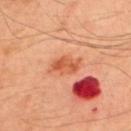workup: no biopsy performed (imaged during a skin exam); illumination: cross-polarized illumination; body site: the upper back; subject: male, aged approximately 50; image-analysis metrics: an area of roughly 6.5 mm² and a shape-asymmetry score of about 0.25 (0 = symmetric); size: ≈3.5 mm; image: ~15 mm crop, total-body skin-cancer survey.